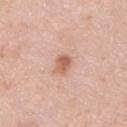From the upper back. A roughly 15 mm field-of-view crop from a total-body skin photograph. Approximately 2.5 mm at its widest. A male subject aged 38–42. The tile uses white-light illumination.